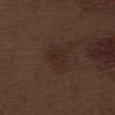Captured during whole-body skin photography for melanoma surveillance; the lesion was not biopsied.
Captured under white-light illumination.
About 2.5 mm across.
Located on the leg.
The patient is a male aged 68–72.
This image is a 15 mm lesion crop taken from a total-body photograph.